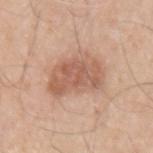Impression: Captured during whole-body skin photography for melanoma surveillance; the lesion was not biopsied. Background: The patient is a male aged around 65. Cropped from a total-body skin-imaging series; the visible field is about 15 mm. Located on the left upper arm.